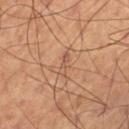workup: imaged on a skin check; not biopsied | patient: male, approximately 70 years of age | location: the leg | tile lighting: cross-polarized | size: about 3 mm | acquisition: 15 mm crop, total-body photography | image-analysis metrics: an area of roughly 2.5 mm², an outline eccentricity of about 0.95 (0 = round, 1 = elongated), and two-axis asymmetry of about 0.55; a lesion color around L≈52 a*≈20 b*≈30 in CIELAB, a lesion–skin lightness drop of about 7, and a normalized border contrast of about 5.5; peripheral color asymmetry of about 0.